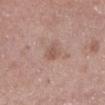{"biopsy_status": "not biopsied; imaged during a skin examination", "patient": {"sex": "male", "age_approx": 75}, "site": "left lower leg", "lighting": "white-light", "automated_metrics": {"cielab_L": 55, "cielab_a": 19, "cielab_b": 25, "vs_skin_darker_L": 8.0, "vs_skin_contrast_norm": 5.5, "border_irregularity_0_10": 3.0, "color_variation_0_10": 1.5, "peripheral_color_asymmetry": 0.5}, "image": {"source": "total-body photography crop", "field_of_view_mm": 15}}